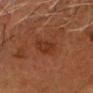Part of a total-body skin-imaging series; this lesion was reviewed on a skin check and was not flagged for biopsy.
The tile uses cross-polarized illumination.
The lesion's longest dimension is about 3.5 mm.
A close-up tile cropped from a whole-body skin photograph, about 15 mm across.
The lesion is on the head or neck.
The subject is a male aged approximately 60.